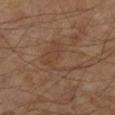notes: imaged on a skin check; not biopsied
patient: male, roughly 65 years of age
acquisition: 15 mm crop, total-body photography
lighting: cross-polarized
lesion size: ≈5 mm